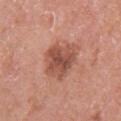Part of a total-body skin-imaging series; this lesion was reviewed on a skin check and was not flagged for biopsy. A close-up tile cropped from a whole-body skin photograph, about 15 mm across. A female subject, aged approximately 50. From the right upper arm.